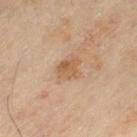{"biopsy_status": "not biopsied; imaged during a skin examination", "site": "left thigh", "image": {"source": "total-body photography crop", "field_of_view_mm": 15}, "lighting": "cross-polarized", "lesion_size": {"long_diameter_mm_approx": 3.0}, "patient": {"sex": "male", "age_approx": 65}}